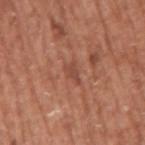{
  "site": "right upper arm",
  "patient": {
    "sex": "male",
    "age_approx": 75
  },
  "image": {
    "source": "total-body photography crop",
    "field_of_view_mm": 15
  }
}